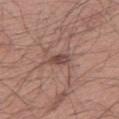workup = total-body-photography surveillance lesion; no biopsy | anatomic site = the right thigh | patient = male, aged around 40 | imaging modality = 15 mm crop, total-body photography.